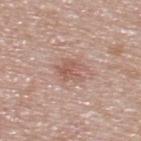{"biopsy_status": "not biopsied; imaged during a skin examination", "lesion_size": {"long_diameter_mm_approx": 3.5}, "patient": {"sex": "male", "age_approx": 55}, "image": {"source": "total-body photography crop", "field_of_view_mm": 15}, "automated_metrics": {"shape_asymmetry": 0.35, "cielab_L": 57, "cielab_a": 21, "cielab_b": 25, "vs_skin_contrast_norm": 6.0, "border_irregularity_0_10": 4.5, "color_variation_0_10": 3.0, "peripheral_color_asymmetry": 1.0, "nevus_likeness_0_100": 0, "lesion_detection_confidence_0_100": 100}, "site": "upper back"}